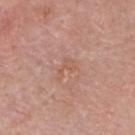| key | value |
|---|---|
| workup | imaged on a skin check; not biopsied |
| anatomic site | the head or neck |
| acquisition | 15 mm crop, total-body photography |
| diameter | ≈3 mm |
| patient | male, roughly 70 years of age |